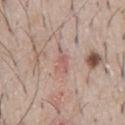The lesion was photographed on a routine skin check and not biopsied; there is no pathology result. The lesion-visualizer software estimated a footprint of about 3.5 mm² and an outline eccentricity of about 0.85 (0 = round, 1 = elongated). The analysis additionally found a within-lesion color-variation index near 2/10 and peripheral color asymmetry of about 0.5. The analysis additionally found a lesion-detection confidence of about 80/100. The patient is a male aged 63 to 67. The tile uses white-light illumination. A 15 mm close-up tile from a total-body photography series done for melanoma screening. The lesion is on the chest.